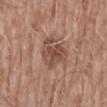Q: Was this lesion biopsied?
A: catalogued during a skin exam; not biopsied
Q: Patient demographics?
A: male, about 60 years old
Q: Lesion size?
A: about 5 mm
Q: Automated lesion metrics?
A: a lesion color around L≈48 a*≈21 b*≈26 in CIELAB, a lesion–skin lightness drop of about 10, and a lesion-to-skin contrast of about 7.5 (normalized; higher = more distinct); a nevus-likeness score of about 0/100 and lesion-presence confidence of about 100/100
Q: What kind of image is this?
A: ~15 mm tile from a whole-body skin photo
Q: Where on the body is the lesion?
A: the abdomen
Q: How was the tile lit?
A: white-light